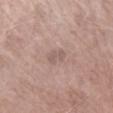Notes:
- notes: imaged on a skin check; not biopsied
- body site: the left forearm
- diameter: about 2.5 mm
- illumination: white-light
- image: 15 mm crop, total-body photography
- subject: female, aged 73–77